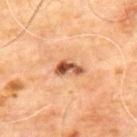workup: total-body-photography surveillance lesion; no biopsy | diameter: about 3.5 mm | patient: male, aged approximately 65 | tile lighting: cross-polarized illumination | automated metrics: an average lesion color of about L≈55 a*≈25 b*≈36 (CIELAB), roughly 16 lightness units darker than nearby skin, and a normalized lesion–skin contrast near 10; a nevus-likeness score of about 85/100 | body site: the upper back | image: ~15 mm tile from a whole-body skin photo.